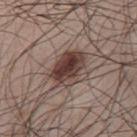This lesion was catalogued during total-body skin photography and was not selected for biopsy.
A roughly 15 mm field-of-view crop from a total-body skin photograph.
The patient is a male aged around 50.
The lesion is located on the upper back.
Measured at roughly 5 mm in maximum diameter.
The tile uses white-light illumination.